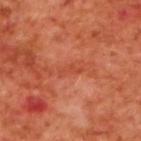Captured during whole-body skin photography for melanoma surveillance; the lesion was not biopsied. About 2.5 mm across. The tile uses cross-polarized illumination. On the upper back. Cropped from a whole-body photographic skin survey; the tile spans about 15 mm. A male subject aged approximately 70.